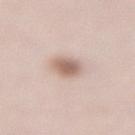Longest diameter approximately 3.5 mm. The patient is a female aged approximately 40. A close-up tile cropped from a whole-body skin photograph, about 15 mm across. The lesion is on the lower back.